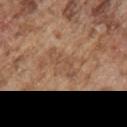Q: Is there a histopathology result?
A: imaged on a skin check; not biopsied
Q: What kind of image is this?
A: total-body-photography crop, ~15 mm field of view
Q: Lesion size?
A: about 4.5 mm
Q: Who is the patient?
A: male, in their mid- to late 70s
Q: Where on the body is the lesion?
A: the right upper arm
Q: How was the tile lit?
A: white-light